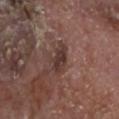biopsy_status: not biopsied; imaged during a skin examination
automated_metrics:
  cielab_L: 36
  cielab_a: 18
  cielab_b: 20
  vs_skin_darker_L: 10.0
  vs_skin_contrast_norm: 8.5
  border_irregularity_0_10: 4.0
  color_variation_0_10: 2.5
  peripheral_color_asymmetry: 1.0
patient:
  sex: male
  age_approx: 80
site: head or neck
lighting: white-light
image:
  source: total-body photography crop
  field_of_view_mm: 15